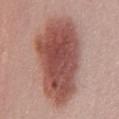Part of a total-body skin-imaging series; this lesion was reviewed on a skin check and was not flagged for biopsy. From the chest. Cropped from a total-body skin-imaging series; the visible field is about 15 mm. The subject is a female aged 48–52.